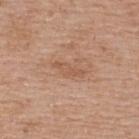From the back.
The patient is a female approximately 60 years of age.
The recorded lesion diameter is about 4 mm.
Imaged with white-light lighting.
A region of skin cropped from a whole-body photographic capture, roughly 15 mm wide.
Automated image analysis of the tile measured an area of roughly 4 mm² and a symmetry-axis asymmetry near 0.4. It also reported a mean CIELAB color near L≈56 a*≈21 b*≈32 and a lesion–skin lightness drop of about 7. The analysis additionally found a border-irregularity rating of about 5/10, a color-variation rating of about 0.5/10, and a peripheral color-asymmetry measure near 0.